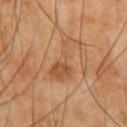Imaged during a routine full-body skin examination; the lesion was not biopsied and no histopathology is available.
Approximately 6 mm at its widest.
Imaged with cross-polarized lighting.
On the chest.
This image is a 15 mm lesion crop taken from a total-body photograph.
The patient is a male in their mid-60s.